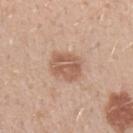<case>
  <biopsy_status>not biopsied; imaged during a skin examination</biopsy_status>
  <lesion_size>
    <long_diameter_mm_approx>3.5</long_diameter_mm_approx>
  </lesion_size>
  <patient>
    <sex>male</sex>
    <age_approx>30</age_approx>
  </patient>
  <lighting>white-light</lighting>
  <image>
    <source>total-body photography crop</source>
    <field_of_view_mm>15</field_of_view_mm>
  </image>
  <site>arm</site>
</case>